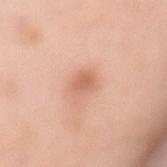Recorded during total-body skin imaging; not selected for excision or biopsy. A lesion tile, about 15 mm wide, cut from a 3D total-body photograph. The patient is a female roughly 50 years of age. Captured under white-light illumination. From the mid back. An algorithmic analysis of the crop reported an area of roughly 4 mm², an outline eccentricity of about 0.8 (0 = round, 1 = elongated), and two-axis asymmetry of about 0.25. The software also gave a border-irregularity rating of about 2.5/10 and radial color variation of about 0.5.